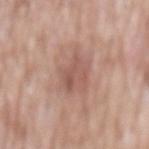notes: total-body-photography surveillance lesion; no biopsy | location: the abdomen | acquisition: ~15 mm crop, total-body skin-cancer survey | subject: male, aged 58–62 | lesion diameter: ~5.5 mm (longest diameter) | tile lighting: white-light.